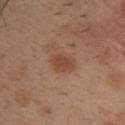Captured during whole-body skin photography for melanoma surveillance; the lesion was not biopsied.